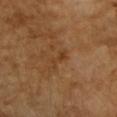Case summary:
• biopsy status · no biopsy performed (imaged during a skin exam)
• acquisition · 15 mm crop, total-body photography
• TBP lesion metrics · a footprint of about 3 mm², a shape eccentricity near 0.9, and a shape-asymmetry score of about 0.45 (0 = symmetric); a border-irregularity index near 6/10 and radial color variation of about 0
• patient · female, aged 68–72
• anatomic site · the right forearm
• illumination · cross-polarized
• lesion diameter · about 3 mm